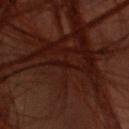Captured under cross-polarized illumination. The lesion is located on the back. A female patient, aged around 40. The lesion-visualizer software estimated an outline eccentricity of about 0.85 (0 = round, 1 = elongated) and a shape-asymmetry score of about 0.3 (0 = symmetric). The analysis additionally found a mean CIELAB color near L≈14 a*≈20 b*≈19, about 4 CIELAB-L* units darker than the surrounding skin, and a lesion-to-skin contrast of about 5.5 (normalized; higher = more distinct). And it measured a border-irregularity rating of about 2.5/10, internal color variation of about 0 on a 0–10 scale, and peripheral color asymmetry of about 0. The software also gave a nevus-likeness score of about 0/100 and a detector confidence of about 0 out of 100 that the crop contains a lesion. Cropped from a total-body skin-imaging series; the visible field is about 15 mm.